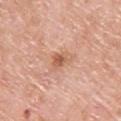{
  "biopsy_status": "not biopsied; imaged during a skin examination",
  "patient": {
    "sex": "male",
    "age_approx": 80
  },
  "image": {
    "source": "total-body photography crop",
    "field_of_view_mm": 15
  },
  "site": "upper back"
}